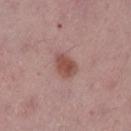Recorded during total-body skin imaging; not selected for excision or biopsy.
A female subject, aged approximately 50.
The lesion's longest dimension is about 3 mm.
This is a white-light tile.
An algorithmic analysis of the crop reported a footprint of about 6 mm², an outline eccentricity of about 0.65 (0 = round, 1 = elongated), and two-axis asymmetry of about 0.25. And it measured about 11 CIELAB-L* units darker than the surrounding skin. It also reported a nevus-likeness score of about 95/100 and a lesion-detection confidence of about 100/100.
A close-up tile cropped from a whole-body skin photograph, about 15 mm across.
From the left lower leg.